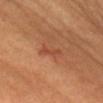  biopsy_status: not biopsied; imaged during a skin examination
  image:
    source: total-body photography crop
    field_of_view_mm: 15
  automated_metrics:
    area_mm2_approx: 2.5
    border_irregularity_0_10: 4.0
    color_variation_0_10: 0.0
    peripheral_color_asymmetry: 0.0
    nevus_likeness_0_100: 10
    lesion_detection_confidence_0_100: 100
  patient:
    sex: female
    age_approx: 60
  lesion_size:
    long_diameter_mm_approx: 2.5
  lighting: cross-polarized
  site: head or neck